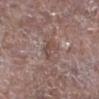This lesion was catalogued during total-body skin photography and was not selected for biopsy.
This is a white-light tile.
An algorithmic analysis of the crop reported a border-irregularity index near 4.5/10, internal color variation of about 3 on a 0–10 scale, and a peripheral color-asymmetry measure near 1.
A male patient, in their mid-60s.
The lesion is located on the right lower leg.
A 15 mm close-up tile from a total-body photography series done for melanoma screening.
Measured at roughly 2.5 mm in maximum diameter.